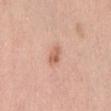body site: the abdomen | acquisition: ~15 mm crop, total-body skin-cancer survey | size: ≈2.5 mm | illumination: white-light | patient: female, aged 38 to 42 | TBP lesion metrics: an area of roughly 4 mm², an outline eccentricity of about 0.7 (0 = round, 1 = elongated), and a shape-asymmetry score of about 0.25 (0 = symmetric); border irregularity of about 2 on a 0–10 scale.